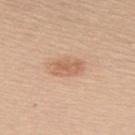biopsy_status: not biopsied; imaged during a skin examination
site: upper back
patient:
  sex: female
  age_approx: 55
image:
  source: total-body photography crop
  field_of_view_mm: 15
lighting: white-light
lesion_size:
  long_diameter_mm_approx: 3.5
automated_metrics:
  area_mm2_approx: 6.0
  eccentricity: 0.85
  shape_asymmetry: 0.2
  cielab_L: 62
  cielab_a: 22
  cielab_b: 32
  vs_skin_darker_L: 10.0
  vs_skin_contrast_norm: 6.0
  border_irregularity_0_10: 2.5
  color_variation_0_10: 2.5
  peripheral_color_asymmetry: 1.0